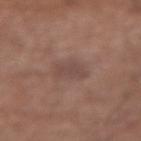– biopsy status: imaged on a skin check; not biopsied
– imaging modality: total-body-photography crop, ~15 mm field of view
– site: the left forearm
– patient: male, aged around 65